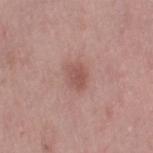Part of a total-body skin-imaging series; this lesion was reviewed on a skin check and was not flagged for biopsy.
The tile uses white-light illumination.
Automated image analysis of the tile measured a classifier nevus-likeness of about 35/100 and a detector confidence of about 100 out of 100 that the crop contains a lesion.
From the right thigh.
A 15 mm close-up extracted from a 3D total-body photography capture.
A female subject, roughly 50 years of age.
Longest diameter approximately 3 mm.